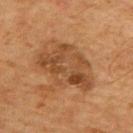This lesion was catalogued during total-body skin photography and was not selected for biopsy. The tile uses cross-polarized illumination. Cropped from a total-body skin-imaging series; the visible field is about 15 mm. Approximately 7 mm at its widest. A male subject about 65 years old. Located on the upper back. An algorithmic analysis of the crop reported a lesion color around L≈38 a*≈18 b*≈31 in CIELAB and a normalized lesion–skin contrast near 7. And it measured a border-irregularity index near 4.5/10 and a color-variation rating of about 5/10.